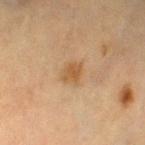notes — catalogued during a skin exam; not biopsied
subject — female, in their mid-50s
lesion size — ~2.5 mm (longest diameter)
imaging modality — total-body-photography crop, ~15 mm field of view
illumination — cross-polarized illumination
body site — the left lower leg